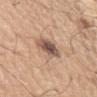Assessment:
No biopsy was performed on this lesion — it was imaged during a full skin examination and was not determined to be concerning.
Clinical summary:
Automated tile analysis of the lesion measured a symmetry-axis asymmetry near 0.2. The software also gave border irregularity of about 3 on a 0–10 scale, a color-variation rating of about 7.5/10, and radial color variation of about 2. The tile uses white-light illumination. The patient is a male in their 60s. About 4 mm across. From the left upper arm. A 15 mm close-up extracted from a 3D total-body photography capture.